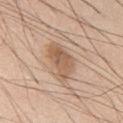Captured during whole-body skin photography for melanoma surveillance; the lesion was not biopsied.
A male patient, roughly 60 years of age.
An algorithmic analysis of the crop reported a shape eccentricity near 0.8 and a shape-asymmetry score of about 0.4 (0 = symmetric). The software also gave a lesion color around L≈59 a*≈18 b*≈31 in CIELAB, about 10 CIELAB-L* units darker than the surrounding skin, and a normalized lesion–skin contrast near 7. The analysis additionally found an automated nevus-likeness rating near 55 out of 100 and lesion-presence confidence of about 100/100.
Cropped from a whole-body photographic skin survey; the tile spans about 15 mm.
On the front of the torso.
This is a white-light tile.